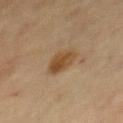Clinical impression: This lesion was catalogued during total-body skin photography and was not selected for biopsy. Image and clinical context: A 15 mm close-up extracted from a 3D total-body photography capture. Longest diameter approximately 3.5 mm. A female subject, aged approximately 60. The total-body-photography lesion software estimated a lesion color around L≈41 a*≈15 b*≈31 in CIELAB. It also reported a classifier nevus-likeness of about 80/100 and lesion-presence confidence of about 100/100. On the chest. The tile uses cross-polarized illumination.